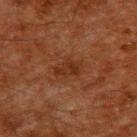Recorded during total-body skin imaging; not selected for excision or biopsy. The recorded lesion diameter is about 3 mm. From the upper back. A male subject aged 58–62. A 15 mm close-up extracted from a 3D total-body photography capture. Automated image analysis of the tile measured border irregularity of about 3 on a 0–10 scale and radial color variation of about 0.5.